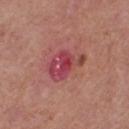subject — female, aged 38–42 | acquisition — 15 mm crop, total-body photography | tile lighting — white-light illumination | image-analysis metrics — a footprint of about 12 mm², an eccentricity of roughly 0.75, and a symmetry-axis asymmetry near 0.3; an average lesion color of about L≈46 a*≈34 b*≈21 (CIELAB) and roughly 10 lightness units darker than nearby skin | anatomic site — the right thigh | lesion diameter — about 5 mm.